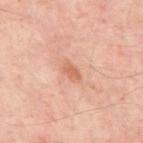Captured during whole-body skin photography for melanoma surveillance; the lesion was not biopsied. The tile uses cross-polarized illumination. The recorded lesion diameter is about 2.5 mm. A close-up tile cropped from a whole-body skin photograph, about 15 mm across. Located on the mid back. A patient about 55 years old.